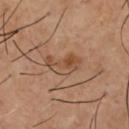Impression: The lesion was tiled from a total-body skin photograph and was not biopsied. Acquisition and patient details: The patient is a male aged around 55. On the chest. The lesion-visualizer software estimated a lesion area of about 7 mm² and an outline eccentricity of about 0.85 (0 = round, 1 = elongated). The software also gave a lesion color around L≈46 a*≈19 b*≈31 in CIELAB, roughly 8 lightness units darker than nearby skin, and a lesion-to-skin contrast of about 6.5 (normalized; higher = more distinct). The software also gave a nevus-likeness score of about 40/100 and a detector confidence of about 100 out of 100 that the crop contains a lesion. A roughly 15 mm field-of-view crop from a total-body skin photograph. The tile uses cross-polarized illumination.